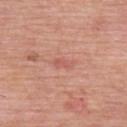The lesion was photographed on a routine skin check and not biopsied; there is no pathology result. Located on the upper back. A male patient, roughly 60 years of age. A region of skin cropped from a whole-body photographic capture, roughly 15 mm wide.